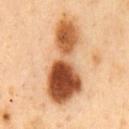{
  "lighting": "cross-polarized",
  "patient": {
    "sex": "male",
    "age_approx": 50
  },
  "lesion_size": {
    "long_diameter_mm_approx": 10.5
  },
  "automated_metrics": {
    "area_mm2_approx": 32.0,
    "shape_asymmetry": 0.4,
    "color_variation_0_10": 10.0,
    "peripheral_color_asymmetry": 5.5
  },
  "image": {
    "source": "total-body photography crop",
    "field_of_view_mm": 15
  },
  "site": "chest"
}